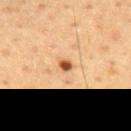Part of a total-body skin-imaging series; this lesion was reviewed on a skin check and was not flagged for biopsy. A male subject, aged 53–57. The lesion is located on the mid back. A region of skin cropped from a whole-body photographic capture, roughly 15 mm wide.